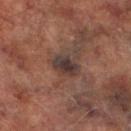{
  "biopsy_status": "not biopsied; imaged during a skin examination",
  "image": {
    "source": "total-body photography crop",
    "field_of_view_mm": 15
  },
  "patient": {
    "sex": "male",
    "age_approx": 75
  },
  "site": "right lower leg"
}